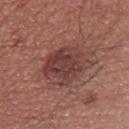Notes:
– notes: imaged on a skin check; not biopsied